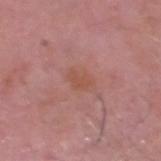notes: total-body-photography surveillance lesion; no biopsy | lighting: white-light | lesion size: ≈3 mm | patient: male, approximately 65 years of age | image-analysis metrics: a shape eccentricity near 0.8 and two-axis asymmetry of about 0.3; a lesion-detection confidence of about 100/100 | acquisition: ~15 mm crop, total-body skin-cancer survey | site: the head or neck.